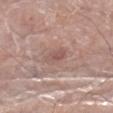Notes:
* notes — imaged on a skin check; not biopsied
* diameter — about 3 mm
* image — ~15 mm crop, total-body skin-cancer survey
* patient — male, aged around 80
* body site — the right lower leg
* lighting — white-light